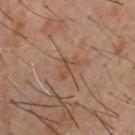The lesion was tiled from a total-body skin photograph and was not biopsied. Captured under cross-polarized illumination. The subject is a male about 60 years old. Located on the chest. The lesion's longest dimension is about 3 mm. A lesion tile, about 15 mm wide, cut from a 3D total-body photograph. Automated image analysis of the tile measured a footprint of about 3.5 mm² and two-axis asymmetry of about 0.45. The analysis additionally found a classifier nevus-likeness of about 0/100 and lesion-presence confidence of about 100/100.